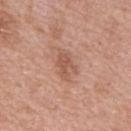Notes:
– workup: imaged on a skin check; not biopsied
– location: the mid back
– acquisition: total-body-photography crop, ~15 mm field of view
– size: ≈3.5 mm
– image-analysis metrics: a footprint of about 5.5 mm² and a symmetry-axis asymmetry near 0.4; about 9 CIELAB-L* units darker than the surrounding skin; a nevus-likeness score of about 5/100 and a lesion-detection confidence of about 100/100
– subject: male, aged 48–52
– tile lighting: white-light illumination